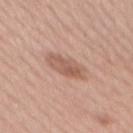Notes:
– notes: catalogued during a skin exam; not biopsied
– patient: female, in their mid- to late 50s
– acquisition: ~15 mm crop, total-body skin-cancer survey
– illumination: white-light illumination
– site: the back
– lesion diameter: ≈5 mm